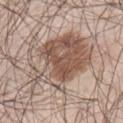Impression:
The lesion was photographed on a routine skin check and not biopsied; there is no pathology result.
Background:
The tile uses white-light illumination. The lesion is on the leg. The recorded lesion diameter is about 8.5 mm. The subject is a male about 70 years old. A region of skin cropped from a whole-body photographic capture, roughly 15 mm wide.